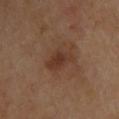Recorded during total-body skin imaging; not selected for excision or biopsy.